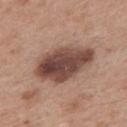Impression: This lesion was catalogued during total-body skin photography and was not selected for biopsy. Image and clinical context: About 7.5 mm across. A female patient roughly 40 years of age. This is a white-light tile. On the back. Automated image analysis of the tile measured a footprint of about 23 mm², a shape eccentricity near 0.85, and a symmetry-axis asymmetry near 0.15. The analysis additionally found an average lesion color of about L≈45 a*≈20 b*≈24 (CIELAB) and roughly 16 lightness units darker than nearby skin. Cropped from a total-body skin-imaging series; the visible field is about 15 mm.